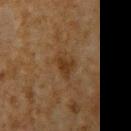notes: no biopsy performed (imaged during a skin exam) | patient: male, aged approximately 60 | imaging modality: 15 mm crop, total-body photography | body site: the left upper arm.